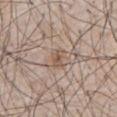follow-up: no biopsy performed (imaged during a skin exam) | patient: male, aged 63 to 67 | tile lighting: white-light illumination | location: the chest | image: ~15 mm crop, total-body skin-cancer survey | diameter: about 3 mm.